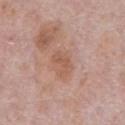Findings:
• follow-up — imaged on a skin check; not biopsied
• image — total-body-photography crop, ~15 mm field of view
• subject — male, aged approximately 70
• tile lighting — white-light
• TBP lesion metrics — a lesion color around L≈56 a*≈22 b*≈29 in CIELAB, roughly 7 lightness units darker than nearby skin, and a normalized border contrast of about 5.5; border irregularity of about 5 on a 0–10 scale, a color-variation rating of about 2/10, and radial color variation of about 0.5
• anatomic site — the chest
• size — ~3 mm (longest diameter)